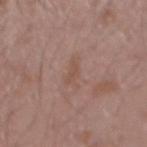biopsy status: catalogued during a skin exam; not biopsied | site: the right forearm | diameter: ~3.5 mm (longest diameter) | subject: male, roughly 20 years of age | image source: ~15 mm crop, total-body skin-cancer survey | image-analysis metrics: a footprint of about 4 mm²; a lesion color around L≈51 a*≈18 b*≈25 in CIELAB, roughly 5 lightness units darker than nearby skin, and a normalized border contrast of about 4.5 | lighting: white-light.